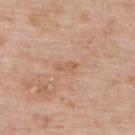subject=male, aged approximately 75; location=the upper back; diameter=about 2.5 mm; automated metrics=a footprint of about 2.5 mm² and a symmetry-axis asymmetry near 0.3; image source=~15 mm crop, total-body skin-cancer survey.